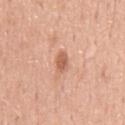workup: imaged on a skin check; not biopsied | patient: male, aged 53 to 57 | image: ~15 mm crop, total-body skin-cancer survey | anatomic site: the chest | lighting: white-light illumination | lesion diameter: ~3 mm (longest diameter).